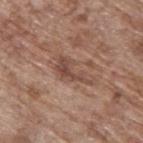The lesion was tiled from a total-body skin photograph and was not biopsied.
This is a white-light tile.
A region of skin cropped from a whole-body photographic capture, roughly 15 mm wide.
From the upper back.
The subject is a male about 70 years old.